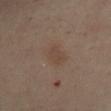– workup — catalogued during a skin exam; not biopsied
– image-analysis metrics — an eccentricity of roughly 0.75 and two-axis asymmetry of about 0.2; an average lesion color of about L≈41 a*≈14 b*≈23 (CIELAB), a lesion–skin lightness drop of about 5, and a normalized lesion–skin contrast near 4.5; an automated nevus-likeness rating near 20 out of 100 and lesion-presence confidence of about 100/100
– tile lighting — cross-polarized
– image source — ~15 mm tile from a whole-body skin photo
– anatomic site — the abdomen
– lesion diameter — ≈3.5 mm
– subject — male, aged 63–67